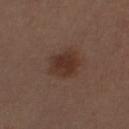notes = catalogued during a skin exam; not biopsied
subject = male, approximately 30 years of age
illumination = white-light
body site = the arm
TBP lesion metrics = a border-irregularity index near 2/10, a within-lesion color-variation index near 2.5/10, and peripheral color asymmetry of about 0.5; a nevus-likeness score of about 95/100 and lesion-presence confidence of about 100/100
lesion size = ~3.5 mm (longest diameter)
acquisition = ~15 mm crop, total-body skin-cancer survey